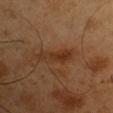Impression: No biopsy was performed on this lesion — it was imaged during a full skin examination and was not determined to be concerning. Clinical summary: A male subject, aged 48–52. About 4.5 mm across. The lesion is on the right upper arm. Imaged with cross-polarized lighting. The total-body-photography lesion software estimated a lesion area of about 6.5 mm² and a shape-asymmetry score of about 0.3 (0 = symmetric). It also reported a detector confidence of about 100 out of 100 that the crop contains a lesion. A region of skin cropped from a whole-body photographic capture, roughly 15 mm wide.